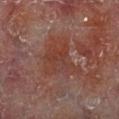Assessment: No biopsy was performed on this lesion — it was imaged during a full skin examination and was not determined to be concerning. Image and clinical context: On the right lower leg. A 15 mm close-up tile from a total-body photography series done for melanoma screening. This is a cross-polarized tile. Automated image analysis of the tile measured an area of roughly 15 mm², a shape eccentricity near 0.6, and two-axis asymmetry of about 0.5. The software also gave a mean CIELAB color near L≈34 a*≈21 b*≈24 and roughly 5 lightness units darker than nearby skin. Measured at roughly 5.5 mm in maximum diameter. A male subject aged 58–62.